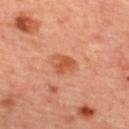{
  "image": {
    "source": "total-body photography crop",
    "field_of_view_mm": 15
  },
  "lighting": "cross-polarized",
  "automated_metrics": {
    "border_irregularity_0_10": 3.5,
    "peripheral_color_asymmetry": 0.5,
    "nevus_likeness_0_100": 70,
    "lesion_detection_confidence_0_100": 100
  },
  "site": "back",
  "lesion_size": {
    "long_diameter_mm_approx": 3.0
  },
  "patient": {
    "sex": "female",
    "age_approx": 45
  }
}